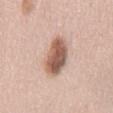Q: Is there a histopathology result?
A: no biopsy performed (imaged during a skin exam)
Q: How was this image acquired?
A: ~15 mm tile from a whole-body skin photo
Q: Patient demographics?
A: female, aged 38 to 42
Q: What lighting was used for the tile?
A: white-light
Q: What is the lesion's diameter?
A: ≈6 mm
Q: Lesion location?
A: the mid back
Q: What did automated image analysis measure?
A: a border-irregularity index near 2.5/10, a color-variation rating of about 6/10, and peripheral color asymmetry of about 2; a nevus-likeness score of about 100/100